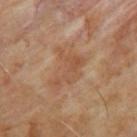Q: How was the tile lit?
A: cross-polarized
Q: Lesion size?
A: ≈4.5 mm
Q: What are the patient's age and sex?
A: male, aged 63–67
Q: What is the imaging modality?
A: total-body-photography crop, ~15 mm field of view
Q: Where on the body is the lesion?
A: the left upper arm